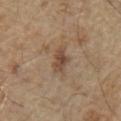Recorded during total-body skin imaging; not selected for excision or biopsy.
The patient is a male aged 68 to 72.
This is a white-light tile.
The recorded lesion diameter is about 3.5 mm.
An algorithmic analysis of the crop reported a border-irregularity rating of about 3.5/10 and a color-variation rating of about 3/10. And it measured an automated nevus-likeness rating near 10 out of 100 and a detector confidence of about 100 out of 100 that the crop contains a lesion.
A close-up tile cropped from a whole-body skin photograph, about 15 mm across.
On the chest.